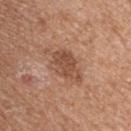<tbp_lesion>
  <biopsy_status>not biopsied; imaged during a skin examination</biopsy_status>
  <patient>
    <sex>female</sex>
    <age_approx>70</age_approx>
  </patient>
  <image>
    <source>total-body photography crop</source>
    <field_of_view_mm>15</field_of_view_mm>
  </image>
  <site>upper back</site>
  <lesion_size>
    <long_diameter_mm_approx>5.0</long_diameter_mm_approx>
  </lesion_size>
</tbp_lesion>